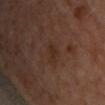Q: Is there a histopathology result?
A: no biopsy performed (imaged during a skin exam)
Q: How was this image acquired?
A: 15 mm crop, total-body photography
Q: How was the tile lit?
A: cross-polarized
Q: Lesion location?
A: the front of the torso
Q: Automated lesion metrics?
A: border irregularity of about 3 on a 0–10 scale, a within-lesion color-variation index near 1.5/10, and a peripheral color-asymmetry measure near 0.5; an automated nevus-likeness rating near 0 out of 100 and a detector confidence of about 100 out of 100 that the crop contains a lesion
Q: Who is the patient?
A: female, in their 60s